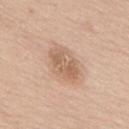{"lighting": "white-light", "lesion_size": {"long_diameter_mm_approx": 5.0}, "site": "upper back", "image": {"source": "total-body photography crop", "field_of_view_mm": 15}, "patient": {"sex": "male", "age_approx": 65}}